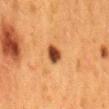The lesion was tiled from a total-body skin photograph and was not biopsied.
A female subject aged 48–52.
This image is a 15 mm lesion crop taken from a total-body photograph.
The tile uses cross-polarized illumination.
The lesion is located on the mid back.
The recorded lesion diameter is about 2.5 mm.